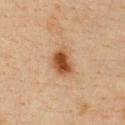Captured during whole-body skin photography for melanoma surveillance; the lesion was not biopsied. Automated image analysis of the tile measured an eccentricity of roughly 0.7 and a symmetry-axis asymmetry near 0.25. The analysis additionally found an average lesion color of about L≈42 a*≈20 b*≈32 (CIELAB) and a lesion–skin lightness drop of about 12. The analysis additionally found a border-irregularity rating of about 2/10, internal color variation of about 5 on a 0–10 scale, and peripheral color asymmetry of about 1.5. Located on the chest. The subject is a female roughly 40 years of age. The recorded lesion diameter is about 3.5 mm. A 15 mm close-up extracted from a 3D total-body photography capture.